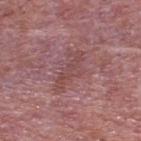Findings:
• follow-up · no biopsy performed (imaged during a skin exam)
• acquisition · ~15 mm crop, total-body skin-cancer survey
• TBP lesion metrics · a lesion area of about 9 mm² and two-axis asymmetry of about 0.35; a nevus-likeness score of about 0/100 and lesion-presence confidence of about 75/100
• patient · male, approximately 75 years of age
• body site · the upper back